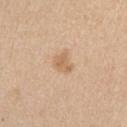| feature | finding |
|---|---|
| biopsy status | catalogued during a skin exam; not biopsied |
| image | total-body-photography crop, ~15 mm field of view |
| patient | female, about 40 years old |
| tile lighting | white-light |
| body site | the right upper arm |